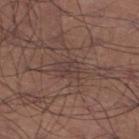Assessment: Imaged during a routine full-body skin examination; the lesion was not biopsied and no histopathology is available. Acquisition and patient details: Captured under white-light illumination. A lesion tile, about 15 mm wide, cut from a 3D total-body photograph. The lesion is on the left lower leg. An algorithmic analysis of the crop reported an outline eccentricity of about 0.6 (0 = round, 1 = elongated) and two-axis asymmetry of about 0.3. The software also gave a border-irregularity index near 3/10, a color-variation rating of about 3/10, and peripheral color asymmetry of about 1. The software also gave a detector confidence of about 65 out of 100 that the crop contains a lesion. About 2.5 mm across. A male patient, aged 43–47.